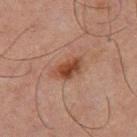Part of a total-body skin-imaging series; this lesion was reviewed on a skin check and was not flagged for biopsy. A male subject, aged approximately 65. The tile uses cross-polarized illumination. A 15 mm close-up extracted from a 3D total-body photography capture. The lesion is located on the right thigh.